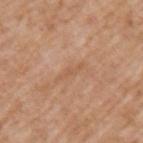Captured during whole-body skin photography for melanoma surveillance; the lesion was not biopsied.
The total-body-photography lesion software estimated a classifier nevus-likeness of about 0/100 and lesion-presence confidence of about 85/100.
Located on the left upper arm.
The tile uses white-light illumination.
The subject is a male aged around 60.
A lesion tile, about 15 mm wide, cut from a 3D total-body photograph.